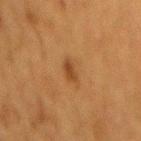The tile uses cross-polarized illumination. Located on the mid back. A 15 mm crop from a total-body photograph taken for skin-cancer surveillance. A female patient aged around 50.